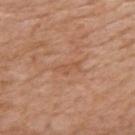Part of a total-body skin-imaging series; this lesion was reviewed on a skin check and was not flagged for biopsy. Imaged with white-light lighting. From the upper back. A region of skin cropped from a whole-body photographic capture, roughly 15 mm wide. A female patient, aged 68–72. About 3 mm across.